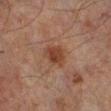biopsy_status: not biopsied; imaged during a skin examination
automated_metrics:
  area_mm2_approx: 6.0
  eccentricity: 0.55
  shape_asymmetry: 0.25
  nevus_likeness_0_100: 75
  lesion_detection_confidence_0_100: 100
patient:
  sex: male
  age_approx: 65
site: left lower leg
lighting: cross-polarized
lesion_size:
  long_diameter_mm_approx: 3.0
image:
  source: total-body photography crop
  field_of_view_mm: 15